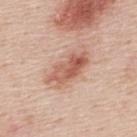Q: Was a biopsy performed?
A: imaged on a skin check; not biopsied
Q: What kind of image is this?
A: ~15 mm crop, total-body skin-cancer survey
Q: Where on the body is the lesion?
A: the back
Q: How was the tile lit?
A: white-light
Q: Who is the patient?
A: male, aged approximately 45
Q: Lesion size?
A: about 5.5 mm